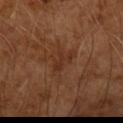This lesion was catalogued during total-body skin photography and was not selected for biopsy. A male patient, in their mid-60s. A 15 mm close-up tile from a total-body photography series done for melanoma screening. This is a cross-polarized tile. Longest diameter approximately 3 mm.